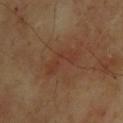follow-up: total-body-photography surveillance lesion; no biopsy | TBP lesion metrics: a footprint of about 7 mm², an outline eccentricity of about 0.95 (0 = round, 1 = elongated), and two-axis asymmetry of about 0.55; roughly 6 lightness units darker than nearby skin and a normalized lesion–skin contrast near 5; border irregularity of about 7 on a 0–10 scale, internal color variation of about 2 on a 0–10 scale, and a peripheral color-asymmetry measure near 0.5; an automated nevus-likeness rating near 0 out of 100 and a detector confidence of about 100 out of 100 that the crop contains a lesion | subject: male, aged 63 to 67 | tile lighting: cross-polarized illumination | lesion size: ~5 mm (longest diameter) | body site: the upper back | acquisition: 15 mm crop, total-body photography.